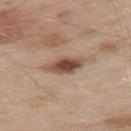notes: total-body-photography surveillance lesion; no biopsy
imaging modality: ~15 mm tile from a whole-body skin photo
patient: male, in their mid- to late 50s
lesion size: ≈5 mm
site: the upper back
TBP lesion metrics: an eccentricity of roughly 0.9 and a shape-asymmetry score of about 0.2 (0 = symmetric); a border-irregularity rating of about 2.5/10 and a within-lesion color-variation index near 5.5/10; an automated nevus-likeness rating near 100 out of 100 and a detector confidence of about 100 out of 100 that the crop contains a lesion